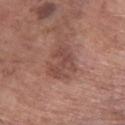Findings:
• notes · imaged on a skin check; not biopsied
• acquisition · total-body-photography crop, ~15 mm field of view
• subject · male, aged 58 to 62
• size · ~4.5 mm (longest diameter)
• automated lesion analysis · a lesion area of about 9.5 mm², an eccentricity of roughly 0.7, and a shape-asymmetry score of about 0.45 (0 = symmetric); an average lesion color of about L≈47 a*≈22 b*≈25 (CIELAB), a lesion–skin lightness drop of about 8, and a normalized border contrast of about 6; a within-lesion color-variation index near 2.5/10 and radial color variation of about 1
• site · the right forearm